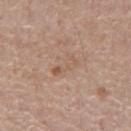follow-up — catalogued during a skin exam; not biopsied
diameter — ≈3.5 mm
acquisition — ~15 mm crop, total-body skin-cancer survey
patient — male, about 55 years old
tile lighting — white-light